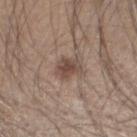The lesion was photographed on a routine skin check and not biopsied; there is no pathology result. Automated tile analysis of the lesion measured a border-irregularity rating of about 3/10, internal color variation of about 3 on a 0–10 scale, and a peripheral color-asymmetry measure near 1. The analysis additionally found a nevus-likeness score of about 85/100 and a detector confidence of about 100 out of 100 that the crop contains a lesion. A male subject roughly 55 years of age. The lesion is located on the right forearm. Cropped from a total-body skin-imaging series; the visible field is about 15 mm. This is a white-light tile.